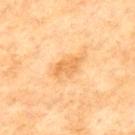Impression:
Imaged during a routine full-body skin examination; the lesion was not biopsied and no histopathology is available.
Clinical summary:
A lesion tile, about 15 mm wide, cut from a 3D total-body photograph. Automated image analysis of the tile measured a lesion color around L≈57 a*≈20 b*≈41 in CIELAB and a lesion–skin lightness drop of about 8. The software also gave border irregularity of about 3.5 on a 0–10 scale, a within-lesion color-variation index near 1.5/10, and radial color variation of about 0.5. Captured under cross-polarized illumination. The lesion's longest dimension is about 3.5 mm. A male patient, aged 68–72. Located on the back.